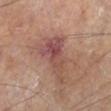Findings:
- follow-up — catalogued during a skin exam; not biopsied
- site — the left lower leg
- lesion diameter — ≈6 mm
- acquisition — total-body-photography crop, ~15 mm field of view
- patient — male, aged around 65